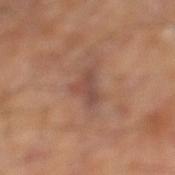Case summary:
* follow-up · total-body-photography surveillance lesion; no biopsy
* image-analysis metrics · a lesion color around L≈48 a*≈21 b*≈27 in CIELAB, about 8 CIELAB-L* units darker than the surrounding skin, and a normalized lesion–skin contrast near 6.5; an automated nevus-likeness rating near 0 out of 100
* site · the left thigh
* size · ~4 mm (longest diameter)
* tile lighting · cross-polarized
* imaging modality · total-body-photography crop, ~15 mm field of view